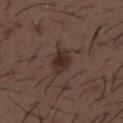Impression: This lesion was catalogued during total-body skin photography and was not selected for biopsy. Context: Located on the mid back. A male subject about 50 years old. Automated image analysis of the tile measured a mean CIELAB color near L≈29 a*≈15 b*≈21, roughly 8 lightness units darker than nearby skin, and a normalized lesion–skin contrast near 8.5. And it measured a border-irregularity rating of about 3/10 and peripheral color asymmetry of about 1. A roughly 15 mm field-of-view crop from a total-body skin photograph.